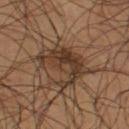Findings:
* workup: imaged on a skin check; not biopsied
* TBP lesion metrics: an average lesion color of about L≈33 a*≈15 b*≈25 (CIELAB), roughly 9 lightness units darker than nearby skin, and a normalized lesion–skin contrast near 8.5; a classifier nevus-likeness of about 15/100 and lesion-presence confidence of about 85/100
* location: the right thigh
* subject: male, in their mid- to late 50s
* lesion size: ~6 mm (longest diameter)
* image source: total-body-photography crop, ~15 mm field of view